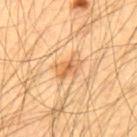No biopsy was performed on this lesion — it was imaged during a full skin examination and was not determined to be concerning. A 15 mm close-up tile from a total-body photography series done for melanoma screening. About 3 mm across. From the upper back. A male subject aged 53–57.